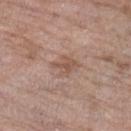Part of a total-body skin-imaging series; this lesion was reviewed on a skin check and was not flagged for biopsy.
Imaged with white-light lighting.
On the left lower leg.
Longest diameter approximately 2.5 mm.
A 15 mm close-up tile from a total-body photography series done for melanoma screening.
A female patient, about 85 years old or older.